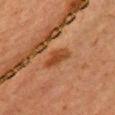Q: Was this lesion biopsied?
A: total-body-photography surveillance lesion; no biopsy
Q: Lesion location?
A: the chest
Q: Automated lesion metrics?
A: an average lesion color of about L≈37 a*≈23 b*≈34 (CIELAB), a lesion–skin lightness drop of about 8, and a normalized lesion–skin contrast near 7.5; a border-irregularity rating of about 3/10, internal color variation of about 2 on a 0–10 scale, and a peripheral color-asymmetry measure near 0.5
Q: What are the patient's age and sex?
A: female, aged approximately 40
Q: What is the imaging modality?
A: ~15 mm crop, total-body skin-cancer survey